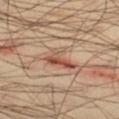The recorded lesion diameter is about 4 mm.
A male patient about 40 years old.
On the right thigh.
Imaged with cross-polarized lighting.
Cropped from a total-body skin-imaging series; the visible field is about 15 mm.